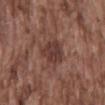Q: Was a biopsy performed?
A: total-body-photography surveillance lesion; no biopsy
Q: Where on the body is the lesion?
A: the back
Q: Patient demographics?
A: male, about 75 years old
Q: What did automated image analysis measure?
A: roughly 9 lightness units darker than nearby skin and a normalized border contrast of about 7.5; a border-irregularity rating of about 2.5/10 and peripheral color asymmetry of about 1
Q: How was this image acquired?
A: 15 mm crop, total-body photography
Q: What lighting was used for the tile?
A: white-light
Q: What is the lesion's diameter?
A: about 4 mm